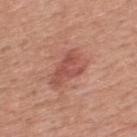Clinical impression:
Imaged during a routine full-body skin examination; the lesion was not biopsied and no histopathology is available.
Acquisition and patient details:
The lesion is on the upper back. An algorithmic analysis of the crop reported a lesion–skin lightness drop of about 9 and a normalized border contrast of about 6.5. About 5 mm across. A male subject aged 53–57. Cropped from a whole-body photographic skin survey; the tile spans about 15 mm.